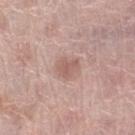Notes:
• notes: catalogued during a skin exam; not biopsied
• automated metrics: an area of roughly 5.5 mm², an outline eccentricity of about 0.55 (0 = round, 1 = elongated), and a shape-asymmetry score of about 0.25 (0 = symmetric); a mean CIELAB color near L≈59 a*≈20 b*≈24, roughly 8 lightness units darker than nearby skin, and a lesion-to-skin contrast of about 5.5 (normalized; higher = more distinct); a border-irregularity rating of about 2.5/10, a within-lesion color-variation index near 1.5/10, and a peripheral color-asymmetry measure near 0.5; a classifier nevus-likeness of about 10/100 and a lesion-detection confidence of about 100/100
• body site: the leg
• lesion size: ~3 mm (longest diameter)
• patient: female, about 60 years old
• tile lighting: white-light illumination
• image source: total-body-photography crop, ~15 mm field of view